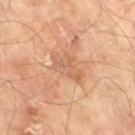Imaged during a routine full-body skin examination; the lesion was not biopsied and no histopathology is available. A 15 mm close-up tile from a total-body photography series done for melanoma screening. Measured at roughly 4 mm in maximum diameter. From the right thigh. Imaged with cross-polarized lighting. A male patient aged 68 to 72.